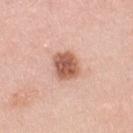{"biopsy_status": "not biopsied; imaged during a skin examination", "patient": {"sex": "female", "age_approx": 35}, "lesion_size": {"long_diameter_mm_approx": 3.5}, "image": {"source": "total-body photography crop", "field_of_view_mm": 15}, "site": "right upper arm"}